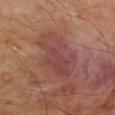Assessment: The lesion was photographed on a routine skin check and not biopsied; there is no pathology result. Image and clinical context: Located on the arm. Cropped from a total-body skin-imaging series; the visible field is about 15 mm. About 6.5 mm across. A male subject, aged around 55. The total-body-photography lesion software estimated a mean CIELAB color near L≈44 a*≈24 b*≈23, a lesion–skin lightness drop of about 6, and a normalized lesion–skin contrast near 5.5. This is a cross-polarized tile.